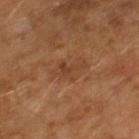Q: Was this lesion biopsied?
A: total-body-photography surveillance lesion; no biopsy
Q: What kind of image is this?
A: ~15 mm crop, total-body skin-cancer survey
Q: Patient demographics?
A: male, about 65 years old